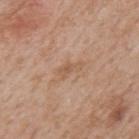Imaged during a routine full-body skin examination; the lesion was not biopsied and no histopathology is available. Captured under white-light illumination. A roughly 15 mm field-of-view crop from a total-body skin photograph. A male subject, approximately 65 years of age. On the mid back.